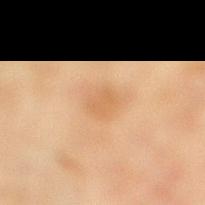<lesion>
  <biopsy_status>not biopsied; imaged during a skin examination</biopsy_status>
  <site>right lower leg</site>
  <image>
    <source>total-body photography crop</source>
    <field_of_view_mm>15</field_of_view_mm>
  </image>
  <patient>
    <sex>female</sex>
    <age_approx>55</age_approx>
  </patient>
  <lesion_size>
    <long_diameter_mm_approx>3.0</long_diameter_mm_approx>
  </lesion_size>
  <lighting>cross-polarized</lighting>
</lesion>